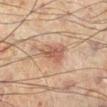{"biopsy_status": "not biopsied; imaged during a skin examination", "image": {"source": "total-body photography crop", "field_of_view_mm": 15}, "lighting": "cross-polarized", "lesion_size": {"long_diameter_mm_approx": 4.0}, "site": "left lower leg", "automated_metrics": {"area_mm2_approx": 8.0, "eccentricity": 0.7, "shape_asymmetry": 0.4, "border_irregularity_0_10": 4.0, "peripheral_color_asymmetry": 1.0, "nevus_likeness_0_100": 45}, "patient": {"sex": "male", "age_approx": 60}}